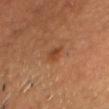The lesion was tiled from a total-body skin photograph and was not biopsied.